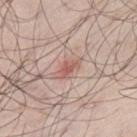The lesion was photographed on a routine skin check and not biopsied; there is no pathology result. The lesion is on the left thigh. A close-up tile cropped from a whole-body skin photograph, about 15 mm across. The subject is a male aged approximately 70. About 3 mm across.